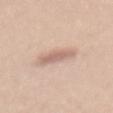Imaged during a routine full-body skin examination; the lesion was not biopsied and no histopathology is available. A 15 mm close-up tile from a total-body photography series done for melanoma screening. On the mid back. The subject is a female roughly 35 years of age.